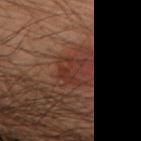notes=imaged on a skin check; not biopsied
tile lighting=cross-polarized illumination
location=the left forearm
imaging modality=15 mm crop, total-body photography
subject=male, about 50 years old
image-analysis metrics=a footprint of about 7.5 mm² and a symmetry-axis asymmetry near 0.35; a border-irregularity index near 4/10, internal color variation of about 3.5 on a 0–10 scale, and peripheral color asymmetry of about 1.5